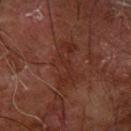Clinical impression: The lesion was tiled from a total-body skin photograph and was not biopsied. Background: The patient is a male roughly 70 years of age. From the left forearm. The total-body-photography lesion software estimated an outline eccentricity of about 0.9 (0 = round, 1 = elongated) and a symmetry-axis asymmetry near 0.4. The software also gave an average lesion color of about L≈23 a*≈19 b*≈21 (CIELAB), a lesion–skin lightness drop of about 4, and a normalized border contrast of about 5. The analysis additionally found a classifier nevus-likeness of about 0/100 and lesion-presence confidence of about 85/100. A 15 mm close-up tile from a total-body photography series done for melanoma screening. Approximately 6 mm at its widest. Imaged with cross-polarized lighting.